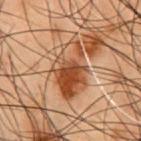The lesion was tiled from a total-body skin photograph and was not biopsied.
The tile uses cross-polarized illumination.
On the chest.
The patient is a male aged 53–57.
Longest diameter approximately 6.5 mm.
Automated image analysis of the tile measured a lesion–skin lightness drop of about 10. The analysis additionally found a border-irregularity index near 2.5/10, a color-variation rating of about 10/10, and radial color variation of about 4.
This image is a 15 mm lesion crop taken from a total-body photograph.